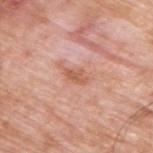Imaged during a routine full-body skin examination; the lesion was not biopsied and no histopathology is available. The lesion is on the arm. Cropped from a whole-body photographic skin survey; the tile spans about 15 mm. A male subject, roughly 60 years of age.